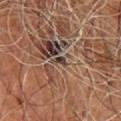follow-up: no biopsy performed (imaged during a skin exam)
patient: male, aged 58 to 62
imaging modality: ~15 mm tile from a whole-body skin photo
body site: the chest
diameter: about 1.5 mm